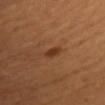<case>
<biopsy_status>not biopsied; imaged during a skin examination</biopsy_status>
<lesion_size>
  <long_diameter_mm_approx>2.5</long_diameter_mm_approx>
</lesion_size>
<site>front of the torso</site>
<image>
  <source>total-body photography crop</source>
  <field_of_view_mm>15</field_of_view_mm>
</image>
<automated_metrics>
  <area_mm2_approx>3.0</area_mm2_approx>
  <eccentricity>0.75</eccentricity>
  <cielab_L>37</cielab_L>
  <cielab_a>24</cielab_a>
  <cielab_b>34</cielab_b>
  <vs_skin_darker_L>9.0</vs_skin_darker_L>
  <vs_skin_contrast_norm>7.5</vs_skin_contrast_norm>
  <border_irregularity_0_10>2.5</border_irregularity_0_10>
  <nevus_likeness_0_100>95</nevus_likeness_0_100>
  <lesion_detection_confidence_0_100>100</lesion_detection_confidence_0_100>
</automated_metrics>
<patient>
  <sex>female</sex>
  <age_approx>50</age_approx>
</patient>
</case>